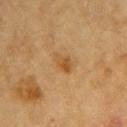The lesion was tiled from a total-body skin photograph and was not biopsied.
Longest diameter approximately 3 mm.
Cropped from a total-body skin-imaging series; the visible field is about 15 mm.
A male subject, aged 83 or over.
The lesion-visualizer software estimated a lesion color around L≈45 a*≈17 b*≈37 in CIELAB, roughly 7 lightness units darker than nearby skin, and a lesion-to-skin contrast of about 6.5 (normalized; higher = more distinct). It also reported a color-variation rating of about 2.5/10 and peripheral color asymmetry of about 1.
This is a cross-polarized tile.
Located on the chest.